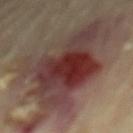Assessment:
Recorded during total-body skin imaging; not selected for excision or biopsy.
Clinical summary:
A male patient roughly 70 years of age. Cropped from a whole-body photographic skin survey; the tile spans about 15 mm. About 11.5 mm across. From the back.